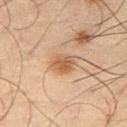This lesion was catalogued during total-body skin photography and was not selected for biopsy.
The tile uses cross-polarized illumination.
The lesion's longest dimension is about 3 mm.
The subject is a male approximately 65 years of age.
Cropped from a whole-body photographic skin survey; the tile spans about 15 mm.
Located on the right thigh.